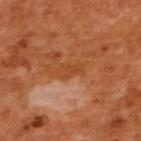<tbp_lesion>
<biopsy_status>not biopsied; imaged during a skin examination</biopsy_status>
</tbp_lesion>